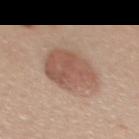biopsy_status: not biopsied; imaged during a skin examination
site: upper back
patient:
  sex: female
  age_approx: 40
automated_metrics:
  area_mm2_approx: 21.0
  shape_asymmetry: 0.15
  cielab_L: 55
  cielab_a: 20
  cielab_b: 27
  vs_skin_darker_L: 11.0
  border_irregularity_0_10: 2.0
  peripheral_color_asymmetry: 1.5
  nevus_likeness_0_100: 80
  lesion_detection_confidence_0_100: 100
lighting: white-light
image:
  source: total-body photography crop
  field_of_view_mm: 15
lesion_size:
  long_diameter_mm_approx: 6.0